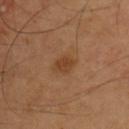Q: Was a biopsy performed?
A: total-body-photography surveillance lesion; no biopsy
Q: What lighting was used for the tile?
A: cross-polarized
Q: Where on the body is the lesion?
A: the chest
Q: Patient demographics?
A: male, approximately 55 years of age
Q: What is the imaging modality?
A: 15 mm crop, total-body photography
Q: Lesion size?
A: about 2.5 mm